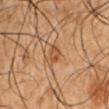workup = no biopsy performed (imaged during a skin exam) | body site = the left upper arm | size = ~3 mm (longest diameter) | image-analysis metrics = a footprint of about 4 mm² and a symmetry-axis asymmetry near 0.3; an average lesion color of about L≈39 a*≈17 b*≈30 (CIELAB), about 7 CIELAB-L* units darker than the surrounding skin, and a normalized border contrast of about 6.5; a classifier nevus-likeness of about 0/100 | acquisition = total-body-photography crop, ~15 mm field of view | illumination = cross-polarized illumination | patient = male, in their 50s.